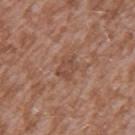Impression: No biopsy was performed on this lesion — it was imaged during a full skin examination and was not determined to be concerning. Clinical summary: The lesion is on the left upper arm. Cropped from a whole-body photographic skin survey; the tile spans about 15 mm. Approximately 3 mm at its widest. A male subject, roughly 45 years of age.